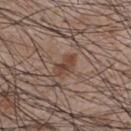Clinical impression: This lesion was catalogued during total-body skin photography and was not selected for biopsy. Background: A close-up tile cropped from a whole-body skin photograph, about 15 mm across. The lesion is on the chest. A male subject about 55 years old. Longest diameter approximately 3.5 mm.